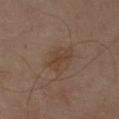Q: Is there a histopathology result?
A: imaged on a skin check; not biopsied
Q: What is the imaging modality?
A: ~15 mm crop, total-body skin-cancer survey
Q: Lesion size?
A: about 3.5 mm
Q: Where on the body is the lesion?
A: the right upper arm
Q: What are the patient's age and sex?
A: male, aged 63–67
Q: Illumination type?
A: cross-polarized